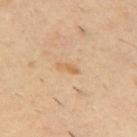The patient is a male aged approximately 40. A 15 mm close-up tile from a total-body photography series done for melanoma screening. Approximately 2.5 mm at its widest. Located on the upper back. The tile uses cross-polarized illumination.